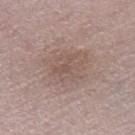* notes — catalogued during a skin exam; not biopsied
* anatomic site — the right thigh
* tile lighting — white-light
* diameter — ≈5.5 mm
* acquisition — ~15 mm crop, total-body skin-cancer survey
* subject — male, aged 63 to 67